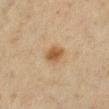No biopsy was performed on this lesion — it was imaged during a full skin examination and was not determined to be concerning. A roughly 15 mm field-of-view crop from a total-body skin photograph. On the left lower leg. Imaged with cross-polarized lighting. A female subject, aged around 50.